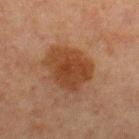The lesion was tiled from a total-body skin photograph and was not biopsied. A 15 mm close-up tile from a total-body photography series done for melanoma screening. Automated tile analysis of the lesion measured an area of roughly 19 mm², a shape eccentricity near 0.65, and two-axis asymmetry of about 0.2. The analysis additionally found a mean CIELAB color near L≈34 a*≈19 b*≈29 and a normalized border contrast of about 8.5. And it measured a border-irregularity index near 2.5/10, a within-lesion color-variation index near 3/10, and radial color variation of about 1. And it measured an automated nevus-likeness rating near 80 out of 100 and a lesion-detection confidence of about 100/100. On the chest. A male patient, about 65 years old. Captured under cross-polarized illumination. Approximately 5.5 mm at its widest.